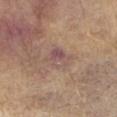Q: Was a biopsy performed?
A: catalogued during a skin exam; not biopsied
Q: What are the patient's age and sex?
A: male, aged approximately 60
Q: How was this image acquired?
A: ~15 mm crop, total-body skin-cancer survey
Q: Where on the body is the lesion?
A: the right forearm